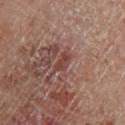<lesion>
<biopsy_status>not biopsied; imaged during a skin examination</biopsy_status>
<automated_metrics>
  <area_mm2_approx>3.0</area_mm2_approx>
  <shape_asymmetry>0.3</shape_asymmetry>
  <nevus_likeness_0_100>0</nevus_likeness_0_100>
  <lesion_detection_confidence_0_100>65</lesion_detection_confidence_0_100>
</automated_metrics>
<lesion_size>
  <long_diameter_mm_approx>2.5</long_diameter_mm_approx>
</lesion_size>
<lighting>cross-polarized</lighting>
<patient>
  <sex>male</sex>
  <age_approx>70</age_approx>
</patient>
<image>
  <source>total-body photography crop</source>
  <field_of_view_mm>15</field_of_view_mm>
</image>
<site>left lower leg</site>
</lesion>